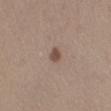This lesion was catalogued during total-body skin photography and was not selected for biopsy.
Captured under white-light illumination.
A region of skin cropped from a whole-body photographic capture, roughly 15 mm wide.
On the leg.
A female subject, in their 40s.